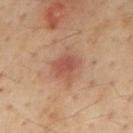follow-up: no biopsy performed (imaged during a skin exam); subject: male, aged 53 to 57; body site: the back; image: ~15 mm tile from a whole-body skin photo; lighting: cross-polarized.